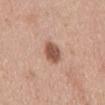Q: What lighting was used for the tile?
A: white-light
Q: Patient demographics?
A: male, about 55 years old
Q: How was this image acquired?
A: 15 mm crop, total-body photography
Q: Where on the body is the lesion?
A: the back
Q: Automated lesion metrics?
A: a lesion area of about 6 mm² and a shape eccentricity near 0.8; a mean CIELAB color near L≈54 a*≈22 b*≈29 and a lesion–skin lightness drop of about 15